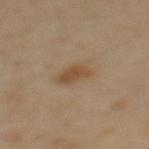Clinical impression: Recorded during total-body skin imaging; not selected for excision or biopsy.